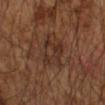Assessment: The lesion was photographed on a routine skin check and not biopsied; there is no pathology result.